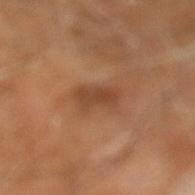<case>
  <biopsy_status>not biopsied; imaged during a skin examination</biopsy_status>
  <image>
    <source>total-body photography crop</source>
    <field_of_view_mm>15</field_of_view_mm>
  </image>
  <site>right leg</site>
  <patient>
    <sex>male</sex>
    <age_approx>60</age_approx>
  </patient>
</case>